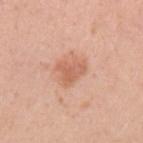Recorded during total-body skin imaging; not selected for excision or biopsy. The lesion is on the left upper arm. This image is a 15 mm lesion crop taken from a total-body photograph. The lesion's longest dimension is about 3.5 mm. The tile uses white-light illumination. A female patient in their 30s. An algorithmic analysis of the crop reported a footprint of about 6.5 mm² and an outline eccentricity of about 0.55 (0 = round, 1 = elongated). The analysis additionally found a mean CIELAB color near L≈62 a*≈25 b*≈32, a lesion–skin lightness drop of about 9, and a normalized lesion–skin contrast near 6. The software also gave a classifier nevus-likeness of about 50/100 and a lesion-detection confidence of about 100/100.